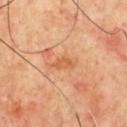<lesion>
  <image>
    <source>total-body photography crop</source>
    <field_of_view_mm>15</field_of_view_mm>
  </image>
  <site>chest</site>
  <patient>
    <sex>male</sex>
    <age_approx>70</age_approx>
  </patient>
</lesion>